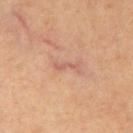The lesion was tiled from a total-body skin photograph and was not biopsied.
An algorithmic analysis of the crop reported a lesion color around L≈57 a*≈23 b*≈28 in CIELAB, a lesion–skin lightness drop of about 8, and a normalized border contrast of about 5.5.
This is a cross-polarized tile.
A 15 mm crop from a total-body photograph taken for skin-cancer surveillance.
The lesion is on the front of the torso.
The subject is a male in their mid-60s.